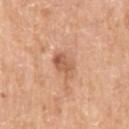biopsy status = total-body-photography surveillance lesion; no biopsy
subject = male, aged 63–67
site = the left upper arm
acquisition = 15 mm crop, total-body photography